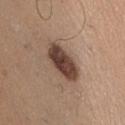notes=total-body-photography surveillance lesion; no biopsy
image source=~15 mm crop, total-body skin-cancer survey
illumination=white-light illumination
automated lesion analysis=a symmetry-axis asymmetry near 0.15; an automated nevus-likeness rating near 75 out of 100 and a lesion-detection confidence of about 100/100
site=the chest
subject=male, in their mid- to late 20s